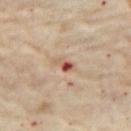Q: Is there a histopathology result?
A: total-body-photography surveillance lesion; no biopsy
Q: What did automated image analysis measure?
A: a lesion–skin lightness drop of about 13; a lesion-detection confidence of about 100/100
Q: Where on the body is the lesion?
A: the right thigh
Q: Who is the patient?
A: female, approximately 60 years of age
Q: What lighting was used for the tile?
A: cross-polarized illumination
Q: How was this image acquired?
A: total-body-photography crop, ~15 mm field of view
Q: What is the lesion's diameter?
A: ≈2.5 mm